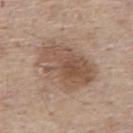{
  "biopsy_status": "not biopsied; imaged during a skin examination",
  "lighting": "white-light",
  "automated_metrics": {
    "border_irregularity_0_10": 3.0,
    "color_variation_0_10": 5.0,
    "peripheral_color_asymmetry": 2.0,
    "nevus_likeness_0_100": 10,
    "lesion_detection_confidence_0_100": 100
  },
  "image": {
    "source": "total-body photography crop",
    "field_of_view_mm": 15
  },
  "patient": {
    "sex": "male",
    "age_approx": 55
  },
  "site": "upper back",
  "lesion_size": {
    "long_diameter_mm_approx": 7.0
  }
}